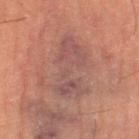image source: ~15 mm crop, total-body skin-cancer survey | illumination: cross-polarized | lesion diameter: ~6.5 mm (longest diameter) | patient: female, approximately 60 years of age | automated metrics: a lesion area of about 25 mm², an outline eccentricity of about 0.75 (0 = round, 1 = elongated), and a shape-asymmetry score of about 0.4 (0 = symmetric); a mean CIELAB color near L≈41 a*≈18 b*≈19, about 5 CIELAB-L* units darker than the surrounding skin, and a normalized border contrast of about 6; a classifier nevus-likeness of about 0/100 and a lesion-detection confidence of about 85/100 | anatomic site: the left thigh.